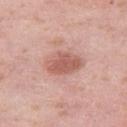This lesion was catalogued during total-body skin photography and was not selected for biopsy. A female patient, aged around 40. Captured under white-light illumination. On the right thigh. A close-up tile cropped from a whole-body skin photograph, about 15 mm across. Approximately 4.5 mm at its widest.